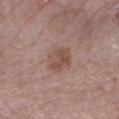notes=imaged on a skin check; not biopsied | patient=male, in their 70s | anatomic site=the right forearm | illumination=white-light illumination | lesion diameter=about 4 mm | acquisition=15 mm crop, total-body photography.